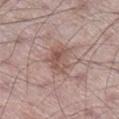Image and clinical context:
A region of skin cropped from a whole-body photographic capture, roughly 15 mm wide. The patient is a male aged 58–62. The lesion is on the right lower leg. The tile uses white-light illumination.